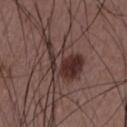Q: Is there a histopathology result?
A: catalogued during a skin exam; not biopsied
Q: How large is the lesion?
A: ~7 mm (longest diameter)
Q: How was the tile lit?
A: white-light illumination
Q: Automated lesion metrics?
A: a mean CIELAB color near L≈32 a*≈17 b*≈19, roughly 10 lightness units darker than nearby skin, and a normalized border contrast of about 9.5; a nevus-likeness score of about 100/100 and lesion-presence confidence of about 100/100
Q: What kind of image is this?
A: 15 mm crop, total-body photography
Q: Patient demographics?
A: male, aged 48–52
Q: Lesion location?
A: the chest